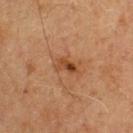Clinical impression: Captured during whole-body skin photography for melanoma surveillance; the lesion was not biopsied. Acquisition and patient details: The total-body-photography lesion software estimated border irregularity of about 3.5 on a 0–10 scale, internal color variation of about 8 on a 0–10 scale, and a peripheral color-asymmetry measure near 3.5. Captured under cross-polarized illumination. Cropped from a whole-body photographic skin survey; the tile spans about 15 mm. A male patient roughly 60 years of age.